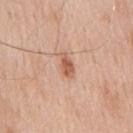Assessment: The lesion was photographed on a routine skin check and not biopsied; there is no pathology result. Image and clinical context: A male subject roughly 60 years of age. Approximately 3 mm at its widest. The total-body-photography lesion software estimated a lesion-detection confidence of about 100/100. The lesion is located on the front of the torso. A 15 mm crop from a total-body photograph taken for skin-cancer surveillance. Captured under white-light illumination.